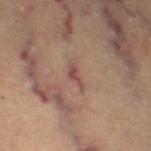No biopsy was performed on this lesion — it was imaged during a full skin examination and was not determined to be concerning. The tile uses cross-polarized illumination. On the left thigh. A subject approximately 60 years of age. Measured at roughly 2.5 mm in maximum diameter. A 15 mm crop from a total-body photograph taken for skin-cancer surveillance.